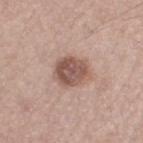This lesion was catalogued during total-body skin photography and was not selected for biopsy. A female subject about 55 years old. Captured under white-light illumination. The recorded lesion diameter is about 4 mm. A close-up tile cropped from a whole-body skin photograph, about 15 mm across. The total-body-photography lesion software estimated a mean CIELAB color near L≈53 a*≈19 b*≈24 and a lesion-to-skin contrast of about 9 (normalized; higher = more distinct). The analysis additionally found a nevus-likeness score of about 20/100 and a lesion-detection confidence of about 100/100. The lesion is located on the left thigh.